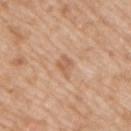Captured during whole-body skin photography for melanoma surveillance; the lesion was not biopsied. The recorded lesion diameter is about 3 mm. A close-up tile cropped from a whole-body skin photograph, about 15 mm across. A male subject roughly 50 years of age. The lesion is on the back. Automated image analysis of the tile measured an average lesion color of about L≈60 a*≈21 b*≈34 (CIELAB) and a normalized border contrast of about 5.5. The analysis additionally found a nevus-likeness score of about 0/100 and lesion-presence confidence of about 100/100. Imaged with white-light lighting.